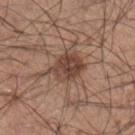Imaged during a routine full-body skin examination; the lesion was not biopsied and no histopathology is available.
Automated image analysis of the tile measured an average lesion color of about L≈43 a*≈19 b*≈26 (CIELAB), roughly 11 lightness units darker than nearby skin, and a normalized lesion–skin contrast near 9. It also reported a nevus-likeness score of about 65/100.
A male patient, about 35 years old.
A roughly 15 mm field-of-view crop from a total-body skin photograph.
On the right lower leg.
Imaged with white-light lighting.
Approximately 4 mm at its widest.